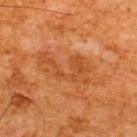This lesion was catalogued during total-body skin photography and was not selected for biopsy.
The patient is a male aged 63 to 67.
The lesion is located on the back.
This image is a 15 mm lesion crop taken from a total-body photograph.